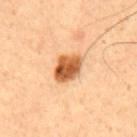Recorded during total-body skin imaging; not selected for excision or biopsy. The lesion-visualizer software estimated a lesion color around L≈56 a*≈26 b*≈41 in CIELAB and roughly 19 lightness units darker than nearby skin. And it measured a nevus-likeness score of about 100/100 and a detector confidence of about 100 out of 100 that the crop contains a lesion. From the mid back. A male subject, aged 48 to 52. Measured at roughly 4 mm in maximum diameter. Captured under cross-polarized illumination. A 15 mm close-up tile from a total-body photography series done for melanoma screening.